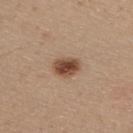| field | value |
|---|---|
| notes | total-body-photography surveillance lesion; no biopsy |
| image source | total-body-photography crop, ~15 mm field of view |
| image-analysis metrics | a detector confidence of about 100 out of 100 that the crop contains a lesion |
| size | about 3.5 mm |
| body site | the right upper arm |
| patient | male, aged approximately 30 |